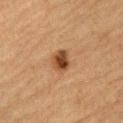Clinical impression: The lesion was tiled from a total-body skin photograph and was not biopsied. Clinical summary: The lesion's longest dimension is about 3 mm. A 15 mm crop from a total-body photograph taken for skin-cancer surveillance. The lesion is on the abdomen. Automated image analysis of the tile measured a shape eccentricity near 0.6. And it measured an average lesion color of about L≈40 a*≈20 b*≈33 (CIELAB), a lesion–skin lightness drop of about 13, and a lesion-to-skin contrast of about 10.5 (normalized; higher = more distinct). And it measured a border-irregularity index near 1.5/10 and a within-lesion color-variation index near 4.5/10. A male patient, about 85 years old or older.